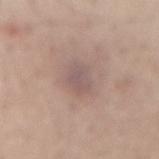<case>
  <biopsy_status>not biopsied; imaged during a skin examination</biopsy_status>
  <site>lower back</site>
  <lighting>white-light</lighting>
  <lesion_size>
    <long_diameter_mm_approx>3.5</long_diameter_mm_approx>
  </lesion_size>
  <patient>
    <sex>male</sex>
    <age_approx>55</age_approx>
  </patient>
  <image>
    <source>total-body photography crop</source>
    <field_of_view_mm>15</field_of_view_mm>
  </image>
  <automated_metrics>
    <eccentricity>0.7</eccentricity>
    <shape_asymmetry>0.25</shape_asymmetry>
    <cielab_L>55</cielab_L>
    <cielab_a>16</cielab_a>
    <cielab_b>20</cielab_b>
    <vs_skin_darker_L>7.0</vs_skin_darker_L>
    <vs_skin_contrast_norm>5.5</vs_skin_contrast_norm>
    <nevus_likeness_0_100>0</nevus_likeness_0_100>
    <lesion_detection_confidence_0_100>100</lesion_detection_confidence_0_100>
  </automated_metrics>
</case>